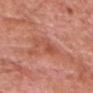• follow-up — total-body-photography surveillance lesion; no biopsy
• image source — ~15 mm tile from a whole-body skin photo
• lesion diameter — ~4.5 mm (longest diameter)
• tile lighting — white-light
• subject — male, about 80 years old
• anatomic site — the head or neck
• automated lesion analysis — an outline eccentricity of about 0.9 (0 = round, 1 = elongated) and two-axis asymmetry of about 0.4; a mean CIELAB color near L≈53 a*≈29 b*≈33 and roughly 7 lightness units darker than nearby skin; a border-irregularity index near 6.5/10, a color-variation rating of about 2/10, and peripheral color asymmetry of about 0.5